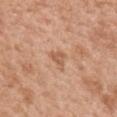subject=female, aged approximately 40
imaging modality=~15 mm crop, total-body skin-cancer survey
TBP lesion metrics=an area of roughly 3.5 mm², an eccentricity of roughly 0.6, and a symmetry-axis asymmetry near 0.5; a mean CIELAB color near L≈58 a*≈22 b*≈34, roughly 9 lightness units darker than nearby skin, and a normalized lesion–skin contrast near 6; a detector confidence of about 100 out of 100 that the crop contains a lesion
site=the mid back
diameter=≈2.5 mm
tile lighting=white-light illumination